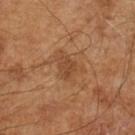Recorded during total-body skin imaging; not selected for excision or biopsy.
A male patient, aged 63–67.
The recorded lesion diameter is about 2.5 mm.
A lesion tile, about 15 mm wide, cut from a 3D total-body photograph.